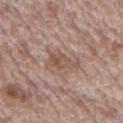Impression: The lesion was photographed on a routine skin check and not biopsied; there is no pathology result. Image and clinical context: Cropped from a total-body skin-imaging series; the visible field is about 15 mm. Imaged with white-light lighting. Located on the mid back. A male subject, aged approximately 70. The total-body-photography lesion software estimated an area of roughly 6.5 mm², an outline eccentricity of about 0.75 (0 = round, 1 = elongated), and a shape-asymmetry score of about 0.45 (0 = symmetric). It also reported border irregularity of about 5 on a 0–10 scale, internal color variation of about 5 on a 0–10 scale, and peripheral color asymmetry of about 2. The recorded lesion diameter is about 4 mm.